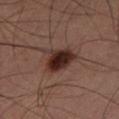Clinical impression: Captured during whole-body skin photography for melanoma surveillance; the lesion was not biopsied. Context: A male subject about 60 years old. The lesion is located on the left lower leg. A 15 mm close-up tile from a total-body photography series done for melanoma screening.